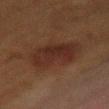Clinical impression: The lesion was tiled from a total-body skin photograph and was not biopsied. Acquisition and patient details: Located on the mid back. A female subject, about 50 years old. A roughly 15 mm field-of-view crop from a total-body skin photograph. Imaged with cross-polarized lighting.